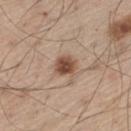imaging modality = 15 mm crop, total-body photography | automated metrics = an area of roughly 5.5 mm², an eccentricity of roughly 0.5, and two-axis asymmetry of about 0.15; a lesion color around L≈49 a*≈19 b*≈28 in CIELAB | lighting = white-light | location = the left thigh | patient = male, roughly 60 years of age.